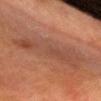This lesion was catalogued during total-body skin photography and was not selected for biopsy. A male subject about 65 years old. A roughly 15 mm field-of-view crop from a total-body skin photograph. The lesion is located on the head or neck.